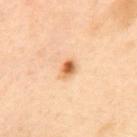biopsy_status: not biopsied; imaged during a skin examination
image:
  source: total-body photography crop
  field_of_view_mm: 15
automated_metrics:
  vs_skin_darker_L: 15.0
  vs_skin_contrast_norm: 9.5
  border_irregularity_0_10: 1.5
  color_variation_0_10: 8.0
  peripheral_color_asymmetry: 2.5
lesion_size:
  long_diameter_mm_approx: 2.5
patient:
  sex: female
  age_approx: 40
lighting: cross-polarized
site: upper back